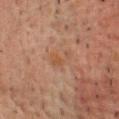{
  "image": {
    "source": "total-body photography crop",
    "field_of_view_mm": 15
  },
  "site": "chest",
  "patient": {
    "sex": "male",
    "age_approx": 60
  },
  "lesion_size": {
    "long_diameter_mm_approx": 3.0
  },
  "lighting": "cross-polarized"
}